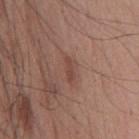* workup: imaged on a skin check; not biopsied
* imaging modality: ~15 mm tile from a whole-body skin photo
* lesion size: ~3 mm (longest diameter)
* illumination: white-light
* body site: the chest
* patient: male, about 55 years old